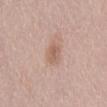Notes:
* workup: catalogued during a skin exam; not biopsied
* illumination: white-light
* lesion diameter: about 2.5 mm
* imaging modality: 15 mm crop, total-body photography
* subject: female, aged approximately 50
* site: the back
* automated metrics: border irregularity of about 2 on a 0–10 scale, a color-variation rating of about 2/10, and peripheral color asymmetry of about 0.5; an automated nevus-likeness rating near 10 out of 100 and a detector confidence of about 100 out of 100 that the crop contains a lesion